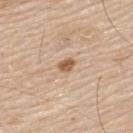follow-up: catalogued during a skin exam; not biopsied | body site: the mid back | subject: male, in their 80s | image source: ~15 mm crop, total-body skin-cancer survey.